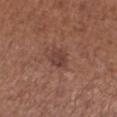{
  "biopsy_status": "not biopsied; imaged during a skin examination",
  "lighting": "white-light",
  "site": "right lower leg",
  "image": {
    "source": "total-body photography crop",
    "field_of_view_mm": 15
  },
  "patient": {
    "sex": "female",
    "age_approx": 55
  },
  "automated_metrics": {
    "area_mm2_approx": 4.5,
    "eccentricity": 0.55,
    "shape_asymmetry": 0.25,
    "border_irregularity_0_10": 2.5,
    "peripheral_color_asymmetry": 1.5,
    "nevus_likeness_0_100": 35,
    "lesion_detection_confidence_0_100": 100
  }
}